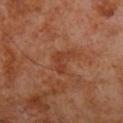Captured under cross-polarized illumination. A male patient, aged around 70. About 3.5 mm across. The lesion is on the leg. A roughly 15 mm field-of-view crop from a total-body skin photograph.